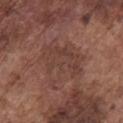Notes:
– biopsy status: no biopsy performed (imaged during a skin exam)
– patient: male, aged 73 to 77
– location: the chest
– TBP lesion metrics: a lesion area of about 19 mm², an eccentricity of roughly 0.2, and a symmetry-axis asymmetry near 0.35; a lesion–skin lightness drop of about 6 and a normalized lesion–skin contrast near 5; a classifier nevus-likeness of about 0/100
– illumination: white-light
– image source: total-body-photography crop, ~15 mm field of view
– diameter: ≈6 mm